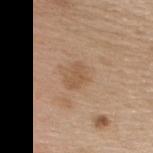The tile uses white-light illumination.
Located on the upper back.
The patient is a female in their mid-40s.
A lesion tile, about 15 mm wide, cut from a 3D total-body photograph.
Measured at roughly 3 mm in maximum diameter.
Automated tile analysis of the lesion measured a lesion area of about 5 mm² and an outline eccentricity of about 0.65 (0 = round, 1 = elongated). It also reported about 7 CIELAB-L* units darker than the surrounding skin and a lesion-to-skin contrast of about 5.5 (normalized; higher = more distinct). It also reported a nevus-likeness score of about 5/100.